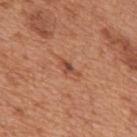follow-up = imaged on a skin check; not biopsied
tile lighting = white-light
anatomic site = the mid back
imaging modality = ~15 mm tile from a whole-body skin photo
image-analysis metrics = an area of roughly 2.5 mm², an eccentricity of roughly 0.9, and two-axis asymmetry of about 0.4
subject = male, aged 63 to 67